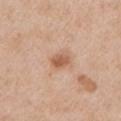Impression: Recorded during total-body skin imaging; not selected for excision or biopsy. Context: A male subject aged 63–67. The tile uses white-light illumination. The total-body-photography lesion software estimated a mean CIELAB color near L≈58 a*≈22 b*≈33, a lesion–skin lightness drop of about 11, and a normalized border contrast of about 7.5. It also reported border irregularity of about 2 on a 0–10 scale, internal color variation of about 2.5 on a 0–10 scale, and radial color variation of about 1. The software also gave a lesion-detection confidence of about 100/100. A 15 mm close-up extracted from a 3D total-body photography capture. The lesion is on the left upper arm.